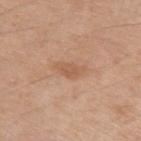The lesion was photographed on a routine skin check and not biopsied; there is no pathology result. The patient is a male in their 60s. This image is a 15 mm lesion crop taken from a total-body photograph. Located on the arm.